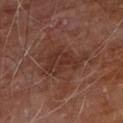Imaged during a routine full-body skin examination; the lesion was not biopsied and no histopathology is available.
A male subject, roughly 60 years of age.
The lesion is on the front of the torso.
Cropped from a whole-body photographic skin survey; the tile spans about 15 mm.
The recorded lesion diameter is about 5.5 mm.
This is a cross-polarized tile.
An algorithmic analysis of the crop reported a mean CIELAB color near L≈30 a*≈20 b*≈24, a lesion–skin lightness drop of about 6, and a normalized border contrast of about 6.5. And it measured a border-irregularity index near 6/10, internal color variation of about 3 on a 0–10 scale, and peripheral color asymmetry of about 1. It also reported an automated nevus-likeness rating near 0 out of 100.